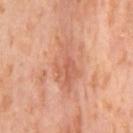{
  "automated_metrics": {
    "cielab_L": 62,
    "cielab_a": 26,
    "cielab_b": 33,
    "vs_skin_darker_L": 9.0,
    "vs_skin_contrast_norm": 5.5,
    "nevus_likeness_0_100": 0,
    "lesion_detection_confidence_0_100": 100
  },
  "lesion_size": {
    "long_diameter_mm_approx": 6.5
  },
  "patient": {
    "sex": "female",
    "age_approx": 55
  },
  "image": {
    "source": "total-body photography crop",
    "field_of_view_mm": 15
  },
  "site": "right thigh",
  "lighting": "cross-polarized"
}